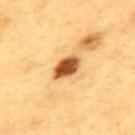Clinical impression:
Part of a total-body skin-imaging series; this lesion was reviewed on a skin check and was not flagged for biopsy.
Image and clinical context:
Automated image analysis of the tile measured an average lesion color of about L≈56 a*≈25 b*≈45 (CIELAB), about 22 CIELAB-L* units darker than the surrounding skin, and a lesion-to-skin contrast of about 13.5 (normalized; higher = more distinct). This is a cross-polarized tile. About 4 mm across. Located on the upper back. A lesion tile, about 15 mm wide, cut from a 3D total-body photograph. A male subject, in their 60s.